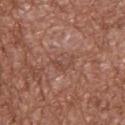Case summary:
- notes · no biopsy performed (imaged during a skin exam)
- lesion size · ~2.5 mm (longest diameter)
- imaging modality · ~15 mm tile from a whole-body skin photo
- subject · male, in their mid- to late 70s
- lighting · white-light
- body site · the upper back